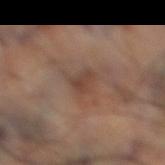No biopsy was performed on this lesion — it was imaged during a full skin examination and was not determined to be concerning. Imaged with cross-polarized lighting. Measured at roughly 3.5 mm in maximum diameter. From the leg. A roughly 15 mm field-of-view crop from a total-body skin photograph.